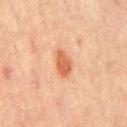No biopsy was performed on this lesion — it was imaged during a full skin examination and was not determined to be concerning. Approximately 3.5 mm at its widest. The lesion is located on the mid back. This image is a 15 mm lesion crop taken from a total-body photograph. An algorithmic analysis of the crop reported a lesion area of about 5.5 mm², an eccentricity of roughly 0.8, and a shape-asymmetry score of about 0.2 (0 = symmetric). The analysis additionally found a lesion-detection confidence of about 100/100. A male patient, in their mid-60s. Imaged with cross-polarized lighting.